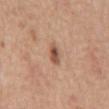Q: Is there a histopathology result?
A: catalogued during a skin exam; not biopsied
Q: Patient demographics?
A: male, roughly 65 years of age
Q: What is the imaging modality?
A: 15 mm crop, total-body photography
Q: What is the anatomic site?
A: the abdomen